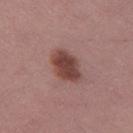notes: total-body-photography surveillance lesion; no biopsy
lesion size: ~4.5 mm (longest diameter)
automated metrics: a mean CIELAB color near L≈43 a*≈22 b*≈22 and a normalized lesion–skin contrast near 10
illumination: white-light
image source: total-body-photography crop, ~15 mm field of view
patient: female, aged approximately 55
site: the leg